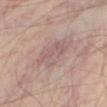The lesion was photographed on a routine skin check and not biopsied; there is no pathology result. A 15 mm close-up tile from a total-body photography series done for melanoma screening. The patient is approximately 55 years of age. From the leg.